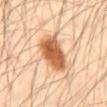Captured during whole-body skin photography for melanoma surveillance; the lesion was not biopsied.
The total-body-photography lesion software estimated a lesion area of about 15 mm² and a shape-asymmetry score of about 0.15 (0 = symmetric). And it measured a mean CIELAB color near L≈58 a*≈23 b*≈37 and about 16 CIELAB-L* units darker than the surrounding skin. The software also gave an automated nevus-likeness rating near 100 out of 100.
A 15 mm close-up extracted from a 3D total-body photography capture.
A male patient in their mid- to late 40s.
The lesion is on the abdomen.
Imaged with cross-polarized lighting.